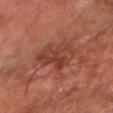{"biopsy_status": "not biopsied; imaged during a skin examination", "automated_metrics": {"area_mm2_approx": 12.0, "eccentricity": 0.8, "shape_asymmetry": 0.3, "border_irregularity_0_10": 4.0, "color_variation_0_10": 4.5, "peripheral_color_asymmetry": 1.5, "nevus_likeness_0_100": 0, "lesion_detection_confidence_0_100": 100}, "site": "right forearm", "lesion_size": {"long_diameter_mm_approx": 5.0}, "lighting": "cross-polarized", "patient": {"age_approx": 65}, "image": {"source": "total-body photography crop", "field_of_view_mm": 15}}